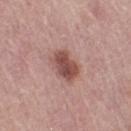The lesion was tiled from a total-body skin photograph and was not biopsied.
The recorded lesion diameter is about 4 mm.
The lesion-visualizer software estimated a lesion color around L≈49 a*≈23 b*≈24 in CIELAB, a lesion–skin lightness drop of about 13, and a normalized lesion–skin contrast near 9.5. The analysis additionally found border irregularity of about 2 on a 0–10 scale and internal color variation of about 4 on a 0–10 scale.
This is a white-light tile.
This image is a 15 mm lesion crop taken from a total-body photograph.
The lesion is located on the leg.
A female subject, aged 63–67.